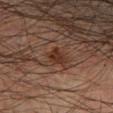<case>
<lighting>cross-polarized</lighting>
<site>right forearm</site>
<image>
  <source>total-body photography crop</source>
  <field_of_view_mm>15</field_of_view_mm>
</image>
<patient>
  <sex>male</sex>
  <age_approx>45</age_approx>
</patient>
</case>